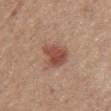biopsy status=catalogued during a skin exam; not biopsied
image=~15 mm crop, total-body skin-cancer survey
image-analysis metrics=a footprint of about 8.5 mm², a shape eccentricity near 0.8, and two-axis asymmetry of about 0.25; a color-variation rating of about 3.5/10 and radial color variation of about 1
subject=female, about 65 years old
size=about 4 mm
tile lighting=white-light
body site=the chest